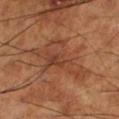Q: Is there a histopathology result?
A: catalogued during a skin exam; not biopsied
Q: How was this image acquired?
A: ~15 mm crop, total-body skin-cancer survey
Q: Who is the patient?
A: male, in their mid-60s
Q: How large is the lesion?
A: ~8 mm (longest diameter)
Q: Where on the body is the lesion?
A: the leg